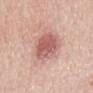No biopsy was performed on this lesion — it was imaged during a full skin examination and was not determined to be concerning.
The lesion's longest dimension is about 5.5 mm.
From the mid back.
An algorithmic analysis of the crop reported a mean CIELAB color near L≈59 a*≈25 b*≈25, about 13 CIELAB-L* units darker than the surrounding skin, and a normalized border contrast of about 8. The analysis additionally found an automated nevus-likeness rating near 80 out of 100.
A male patient, aged 53 to 57.
A close-up tile cropped from a whole-body skin photograph, about 15 mm across.
This is a white-light tile.